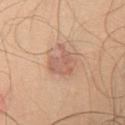Impression:
This lesion was catalogued during total-body skin photography and was not selected for biopsy.
Clinical summary:
The lesion is located on the chest. An algorithmic analysis of the crop reported a lesion color around L≈61 a*≈21 b*≈30 in CIELAB, roughly 9 lightness units darker than nearby skin, and a normalized lesion–skin contrast near 6. The software also gave radial color variation of about 1. A 15 mm crop from a total-body photograph taken for skin-cancer surveillance. The recorded lesion diameter is about 4 mm. Captured under white-light illumination. A male subject, about 45 years old.